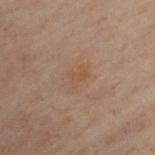– workup · total-body-photography surveillance lesion; no biopsy
– site · the chest
– acquisition · 15 mm crop, total-body photography
– patient · male, in their 50s